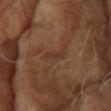Context: The lesion's longest dimension is about 3 mm. Located on the head or neck. A female subject, aged around 75. Cropped from a total-body skin-imaging series; the visible field is about 15 mm.